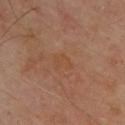{
  "biopsy_status": "not biopsied; imaged during a skin examination",
  "site": "back",
  "lesion_size": {
    "long_diameter_mm_approx": 2.5
  },
  "patient": {
    "sex": "male",
    "age_approx": 65
  },
  "lighting": "cross-polarized",
  "automated_metrics": {
    "area_mm2_approx": 3.5,
    "eccentricity": 0.75,
    "shape_asymmetry": 0.45,
    "cielab_L": 47,
    "cielab_a": 21,
    "cielab_b": 33,
    "vs_skin_darker_L": 4.0,
    "vs_skin_contrast_norm": 4.5,
    "border_irregularity_0_10": 5.5,
    "color_variation_0_10": 0.0,
    "peripheral_color_asymmetry": 0.0,
    "nevus_likeness_0_100": 0
  },
  "image": {
    "source": "total-body photography crop",
    "field_of_view_mm": 15
  }
}